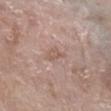follow-up: catalogued during a skin exam; not biopsied
patient: female, aged 73–77
illumination: white-light
diameter: ≈2.5 mm
imaging modality: ~15 mm tile from a whole-body skin photo
anatomic site: the right lower leg
automated metrics: a footprint of about 3 mm², a shape eccentricity near 0.8, and two-axis asymmetry of about 0.45; an average lesion color of about L≈56 a*≈18 b*≈25 (CIELAB) and roughly 7 lightness units darker than nearby skin; a border-irregularity rating of about 4/10, a within-lesion color-variation index near 1/10, and a peripheral color-asymmetry measure near 0.5